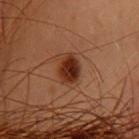| feature | finding |
|---|---|
| notes | total-body-photography surveillance lesion; no biopsy |
| image | ~15 mm crop, total-body skin-cancer survey |
| automated lesion analysis | a lesion area of about 7 mm² and a shape eccentricity near 0.75; an automated nevus-likeness rating near 95 out of 100 and lesion-presence confidence of about 100/100 |
| lesion diameter | ~3.5 mm (longest diameter) |
| patient | female, in their 40s |
| illumination | cross-polarized |
| body site | the upper back |